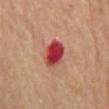Part of a total-body skin-imaging series; this lesion was reviewed on a skin check and was not flagged for biopsy.
Located on the chest.
A male patient, aged around 85 or older.
This is a cross-polarized tile.
This image is a 15 mm lesion crop taken from a total-body photograph.